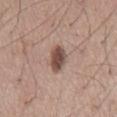Impression:
Imaged during a routine full-body skin examination; the lesion was not biopsied and no histopathology is available.
Background:
Cropped from a total-body skin-imaging series; the visible field is about 15 mm. A male subject in their mid-50s. Automated tile analysis of the lesion measured an average lesion color of about L≈48 a*≈18 b*≈23 (CIELAB), about 15 CIELAB-L* units darker than the surrounding skin, and a lesion-to-skin contrast of about 10.5 (normalized; higher = more distinct). The software also gave a border-irregularity index near 2.5/10. The software also gave a classifier nevus-likeness of about 95/100 and a detector confidence of about 100 out of 100 that the crop contains a lesion. Imaged with white-light lighting. The lesion's longest dimension is about 3.5 mm. Located on the mid back.